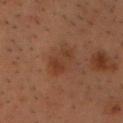Captured during whole-body skin photography for melanoma surveillance; the lesion was not biopsied. A roughly 15 mm field-of-view crop from a total-body skin photograph. This is a cross-polarized tile. An algorithmic analysis of the crop reported an area of roughly 6 mm², an outline eccentricity of about 0.8 (0 = round, 1 = elongated), and a shape-asymmetry score of about 0.3 (0 = symmetric). It also reported a mean CIELAB color near L≈30 a*≈18 b*≈25, roughly 5 lightness units darker than nearby skin, and a normalized border contrast of about 6. From the chest. A male patient, aged approximately 55. The lesion's longest dimension is about 3.5 mm.